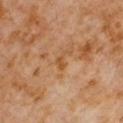Impression: This lesion was catalogued during total-body skin photography and was not selected for biopsy. Clinical summary: Measured at roughly 2.5 mm in maximum diameter. A region of skin cropped from a whole-body photographic capture, roughly 15 mm wide. The lesion is located on the right upper arm. The lesion-visualizer software estimated an outline eccentricity of about 0.85 (0 = round, 1 = elongated) and two-axis asymmetry of about 0.6. It also reported a lesion color around L≈48 a*≈21 b*≈37 in CIELAB, about 6 CIELAB-L* units darker than the surrounding skin, and a lesion-to-skin contrast of about 6 (normalized; higher = more distinct). A male subject about 60 years old. Imaged with cross-polarized lighting.